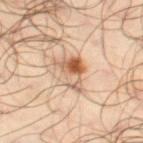Q: Is there a histopathology result?
A: imaged on a skin check; not biopsied
Q: How large is the lesion?
A: about 5 mm
Q: What is the imaging modality?
A: ~15 mm tile from a whole-body skin photo
Q: Automated lesion metrics?
A: an area of roughly 9 mm², a shape eccentricity near 0.8, and a shape-asymmetry score of about 0.55 (0 = symmetric); a mean CIELAB color near L≈58 a*≈19 b*≈32, roughly 13 lightness units darker than nearby skin, and a lesion-to-skin contrast of about 8.5 (normalized; higher = more distinct); a border-irregularity rating of about 6/10, a within-lesion color-variation index near 10/10, and peripheral color asymmetry of about 4.5; lesion-presence confidence of about 100/100
Q: What is the anatomic site?
A: the leg
Q: Who is the patient?
A: male, aged approximately 65
Q: What lighting was used for the tile?
A: cross-polarized illumination